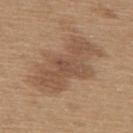Part of a total-body skin-imaging series; this lesion was reviewed on a skin check and was not flagged for biopsy. Measured at roughly 8.5 mm in maximum diameter. A male subject, in their mid-60s. Located on the upper back. The tile uses white-light illumination. A 15 mm close-up extracted from a 3D total-body photography capture.